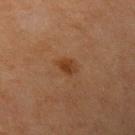• workup — imaged on a skin check; not biopsied
• image source — ~15 mm crop, total-body skin-cancer survey
• location — the left upper arm
• size — ≈2.5 mm
• lighting — cross-polarized
• subject — female, about 65 years old
• image-analysis metrics — a lesion area of about 3.5 mm², an eccentricity of roughly 0.7, and a shape-asymmetry score of about 0.25 (0 = symmetric); a mean CIELAB color near L≈33 a*≈20 b*≈30 and roughly 7 lightness units darker than nearby skin; border irregularity of about 2.5 on a 0–10 scale, a within-lesion color-variation index near 2/10, and a peripheral color-asymmetry measure near 1